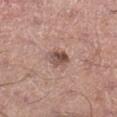<tbp_lesion>
<biopsy_status>not biopsied; imaged during a skin examination</biopsy_status>
<lesion_size>
  <long_diameter_mm_approx>3.0</long_diameter_mm_approx>
</lesion_size>
<patient>
  <sex>male</sex>
  <age_approx>55</age_approx>
</patient>
<site>right lower leg</site>
<image>
  <source>total-body photography crop</source>
  <field_of_view_mm>15</field_of_view_mm>
</image>
<lighting>white-light</lighting>
</tbp_lesion>